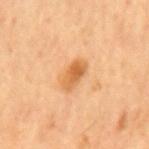The lesion was photographed on a routine skin check and not biopsied; there is no pathology result.
The total-body-photography lesion software estimated a lesion color around L≈63 a*≈26 b*≈45 in CIELAB and a normalized lesion–skin contrast near 8.
A male subject in their mid-60s.
Captured under cross-polarized illumination.
Measured at roughly 3.5 mm in maximum diameter.
A 15 mm close-up extracted from a 3D total-body photography capture.
The lesion is on the mid back.